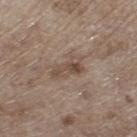Case summary:
* biopsy status: total-body-photography surveillance lesion; no biopsy
* imaging modality: 15 mm crop, total-body photography
* tile lighting: white-light illumination
* body site: the right lower leg
* patient: male, roughly 70 years of age
* TBP lesion metrics: a lesion area of about 5.5 mm², an eccentricity of roughly 0.9, and a shape-asymmetry score of about 0.35 (0 = symmetric); border irregularity of about 4 on a 0–10 scale and a peripheral color-asymmetry measure near 1.5; an automated nevus-likeness rating near 0 out of 100 and lesion-presence confidence of about 100/100
* diameter: about 3.5 mm